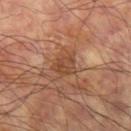biopsy status — catalogued during a skin exam; not biopsied
subject — male, aged 58 to 62
lesion size — about 3 mm
illumination — cross-polarized illumination
image source — ~15 mm tile from a whole-body skin photo
location — the left thigh